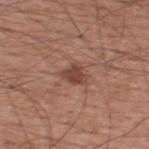The lesion was tiled from a total-body skin photograph and was not biopsied. A 15 mm crop from a total-body photograph taken for skin-cancer surveillance. Measured at roughly 2.5 mm in maximum diameter. The lesion is located on the back. The subject is a male aged 58–62. The tile uses white-light illumination.